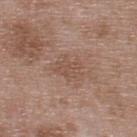– workup: total-body-photography surveillance lesion; no biopsy
– illumination: white-light illumination
– acquisition: 15 mm crop, total-body photography
– TBP lesion metrics: a lesion area of about 5.5 mm², a shape eccentricity near 0.65, and two-axis asymmetry of about 0.3; a lesion color around L≈51 a*≈19 b*≈26 in CIELAB, about 6 CIELAB-L* units darker than the surrounding skin, and a normalized border contrast of about 5; a border-irregularity rating of about 3.5/10, a color-variation rating of about 1.5/10, and a peripheral color-asymmetry measure near 0.5
– lesion size: ≈3 mm
– patient: male, in their 50s
– body site: the upper back